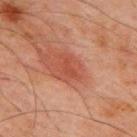{"biopsy_status": "not biopsied; imaged during a skin examination", "image": {"source": "total-body photography crop", "field_of_view_mm": 15}, "automated_metrics": {"area_mm2_approx": 3.0, "eccentricity": 0.85, "shape_asymmetry": 0.5}, "patient": {"sex": "male", "age_approx": 70}, "site": "front of the torso"}